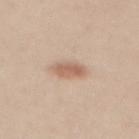Impression:
Recorded during total-body skin imaging; not selected for excision or biopsy.
Clinical summary:
The patient is a female aged 28 to 32. Located on the mid back. The recorded lesion diameter is about 3.5 mm. This is a white-light tile. The total-body-photography lesion software estimated a footprint of about 6 mm² and two-axis asymmetry of about 0.15. And it measured a mean CIELAB color near L≈62 a*≈19 b*≈29, roughly 10 lightness units darker than nearby skin, and a normalized border contrast of about 7. The software also gave a nevus-likeness score of about 95/100 and a detector confidence of about 100 out of 100 that the crop contains a lesion. Cropped from a total-body skin-imaging series; the visible field is about 15 mm.